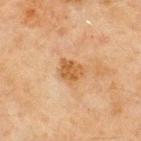| feature | finding |
|---|---|
| workup | total-body-photography surveillance lesion; no biopsy |
| acquisition | ~15 mm tile from a whole-body skin photo |
| subject | male, roughly 45 years of age |
| automated metrics | a lesion–skin lightness drop of about 9 and a lesion-to-skin contrast of about 8 (normalized; higher = more distinct); border irregularity of about 2 on a 0–10 scale, internal color variation of about 2.5 on a 0–10 scale, and peripheral color asymmetry of about 1; a nevus-likeness score of about 10/100 and a detector confidence of about 100 out of 100 that the crop contains a lesion |
| site | the upper back |